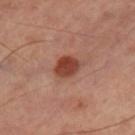The total-body-photography lesion software estimated a classifier nevus-likeness of about 100/100 and a detector confidence of about 100 out of 100 that the crop contains a lesion. This is a cross-polarized tile. The lesion is on the right thigh. A 15 mm close-up tile from a total-body photography series done for melanoma screening.